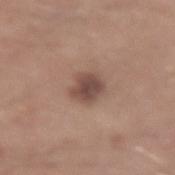workup — no biopsy performed (imaged during a skin exam)
patient — male, aged 23 to 27
anatomic site — the arm
tile lighting — white-light illumination
automated lesion analysis — a lesion area of about 7 mm², a shape eccentricity near 0.45, and a symmetry-axis asymmetry near 0.25; a lesion color around L≈47 a*≈18 b*≈24 in CIELAB and a lesion–skin lightness drop of about 12; a nevus-likeness score of about 65/100 and a lesion-detection confidence of about 100/100
acquisition — 15 mm crop, total-body photography